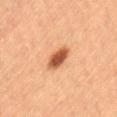Findings:
– workup · total-body-photography surveillance lesion; no biopsy
– illumination · cross-polarized
– TBP lesion metrics · an area of roughly 6.5 mm², an outline eccentricity of about 0.75 (0 = round, 1 = elongated), and two-axis asymmetry of about 0.15; roughly 17 lightness units darker than nearby skin; a border-irregularity rating of about 1.5/10 and radial color variation of about 1.5
– site · the left thigh
– patient · female, aged 58–62
– diameter · ≈3.5 mm
– image source · ~15 mm crop, total-body skin-cancer survey